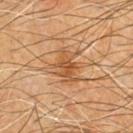This lesion was catalogued during total-body skin photography and was not selected for biopsy. A 15 mm crop from a total-body photograph taken for skin-cancer surveillance. On the front of the torso. A male subject, aged around 60. Approximately 4 mm at its widest.